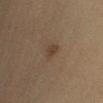Imaged during a routine full-body skin examination; the lesion was not biopsied and no histopathology is available. The recorded lesion diameter is about 2.5 mm. This is a cross-polarized tile. Located on the right lower leg. A female patient, aged approximately 40. A 15 mm close-up tile from a total-body photography series done for melanoma screening.